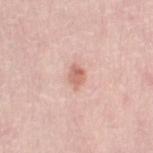Findings:
- notes: catalogued during a skin exam; not biopsied
- diameter: ≈2.5 mm
- image-analysis metrics: an outline eccentricity of about 0.65 (0 = round, 1 = elongated) and a shape-asymmetry score of about 0.2 (0 = symmetric)
- patient: female, approximately 40 years of age
- acquisition: 15 mm crop, total-body photography
- lighting: white-light illumination
- anatomic site: the right thigh